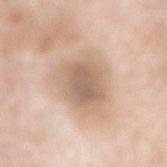Impression: Imaged during a routine full-body skin examination; the lesion was not biopsied and no histopathology is available. Clinical summary: This image is a 15 mm lesion crop taken from a total-body photograph. Located on the mid back. The subject is a male about 80 years old. The recorded lesion diameter is about 6.5 mm.